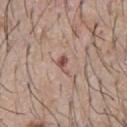workup: catalogued during a skin exam; not biopsied | lesion size: ~2.5 mm (longest diameter) | patient: male, about 65 years old | lighting: white-light illumination | body site: the chest | image: 15 mm crop, total-body photography | automated metrics: an automated nevus-likeness rating near 0 out of 100 and a detector confidence of about 100 out of 100 that the crop contains a lesion.